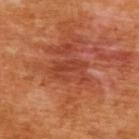Recorded during total-body skin imaging; not selected for excision or biopsy. Longest diameter approximately 4 mm. A female patient approximately 55 years of age. The lesion is on the back. A lesion tile, about 15 mm wide, cut from a 3D total-body photograph. The tile uses cross-polarized illumination. Automated image analysis of the tile measured an area of roughly 7.5 mm², an outline eccentricity of about 0.85 (0 = round, 1 = elongated), and a shape-asymmetry score of about 0.45 (0 = symmetric). The analysis additionally found a mean CIELAB color near L≈45 a*≈32 b*≈35, roughly 8 lightness units darker than nearby skin, and a normalized lesion–skin contrast near 6. The analysis additionally found a nevus-likeness score of about 0/100 and a detector confidence of about 100 out of 100 that the crop contains a lesion.